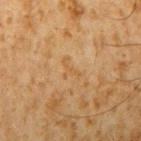- notes · total-body-photography surveillance lesion; no biopsy
- body site · the arm
- image · ~15 mm crop, total-body skin-cancer survey
- diameter · ≈2.5 mm
- subject · male, aged 58 to 62